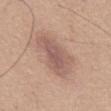Part of a total-body skin-imaging series; this lesion was reviewed on a skin check and was not flagged for biopsy.
Captured under white-light illumination.
Automated tile analysis of the lesion measured an area of roughly 16 mm² and a shape eccentricity near 0.8. And it measured a mean CIELAB color near L≈57 a*≈19 b*≈24, about 9 CIELAB-L* units darker than the surrounding skin, and a normalized border contrast of about 6.5. The analysis additionally found a border-irregularity rating of about 4/10, a within-lesion color-variation index near 3.5/10, and radial color variation of about 1. And it measured lesion-presence confidence of about 100/100.
A region of skin cropped from a whole-body photographic capture, roughly 15 mm wide.
From the mid back.
The recorded lesion diameter is about 5.5 mm.
The patient is a male aged 63 to 67.